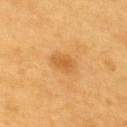This lesion was catalogued during total-body skin photography and was not selected for biopsy.
Automated tile analysis of the lesion measured a normalized border contrast of about 6. The analysis additionally found border irregularity of about 1.5 on a 0–10 scale, a within-lesion color-variation index near 2/10, and peripheral color asymmetry of about 0.5. And it measured a classifier nevus-likeness of about 35/100.
Imaged with cross-polarized lighting.
A lesion tile, about 15 mm wide, cut from a 3D total-body photograph.
Located on the upper back.
Approximately 3 mm at its widest.
A male subject roughly 60 years of age.